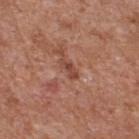Assessment: No biopsy was performed on this lesion — it was imaged during a full skin examination and was not determined to be concerning. Acquisition and patient details: A male patient in their mid- to late 60s. The lesion's longest dimension is about 2.5 mm. Imaged with white-light lighting. A lesion tile, about 15 mm wide, cut from a 3D total-body photograph. From the upper back.